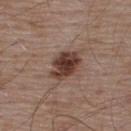Imaged during a routine full-body skin examination; the lesion was not biopsied and no histopathology is available. Captured under white-light illumination. A male patient, in their mid-50s. This image is a 15 mm lesion crop taken from a total-body photograph. From the upper back.